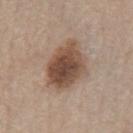notes: catalogued during a skin exam; not biopsied
location: the chest
patient: male, about 65 years old
lighting: white-light
acquisition: ~15 mm crop, total-body skin-cancer survey